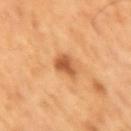Clinical summary:
A male subject aged approximately 60. Cropped from a whole-body photographic skin survey; the tile spans about 15 mm. The lesion is located on the right upper arm.